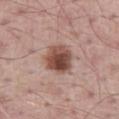{
  "biopsy_status": "not biopsied; imaged during a skin examination",
  "automated_metrics": {
    "area_mm2_approx": 11.0,
    "eccentricity": 0.4,
    "shape_asymmetry": 0.2,
    "vs_skin_darker_L": 15.0,
    "vs_skin_contrast_norm": 10.5,
    "border_irregularity_0_10": 1.5,
    "peripheral_color_asymmetry": 2.5,
    "nevus_likeness_0_100": 95,
    "lesion_detection_confidence_0_100": 100
  },
  "patient": {
    "sex": "male",
    "age_approx": 65
  },
  "site": "abdomen",
  "image": {
    "source": "total-body photography crop",
    "field_of_view_mm": 15
  },
  "lighting": "white-light"
}